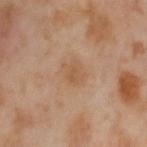Q: Is there a histopathology result?
A: no biopsy performed (imaged during a skin exam)
Q: How was this image acquired?
A: ~15 mm crop, total-body skin-cancer survey
Q: Lesion location?
A: the left thigh
Q: How was the tile lit?
A: cross-polarized
Q: Lesion size?
A: about 3 mm
Q: Who is the patient?
A: female, aged around 55